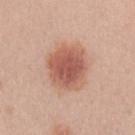The lesion was photographed on a routine skin check and not biopsied; there is no pathology result.